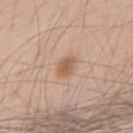Impression:
Captured during whole-body skin photography for melanoma surveillance; the lesion was not biopsied.
Acquisition and patient details:
The subject is a male approximately 70 years of age. Approximately 3 mm at its widest. On the upper back. Cropped from a whole-body photographic skin survey; the tile spans about 15 mm. This is a white-light tile.